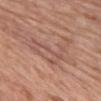<lesion>
<biopsy_status>not biopsied; imaged during a skin examination</biopsy_status>
<lighting>white-light</lighting>
<site>front of the torso</site>
<image>
  <source>total-body photography crop</source>
  <field_of_view_mm>15</field_of_view_mm>
</image>
<lesion_size>
  <long_diameter_mm_approx>7.5</long_diameter_mm_approx>
</lesion_size>
<automated_metrics>
  <vs_skin_darker_L>7.0</vs_skin_darker_L>
  <vs_skin_contrast_norm>5.0</vs_skin_contrast_norm>
  <lesion_detection_confidence_0_100>50</lesion_detection_confidence_0_100>
</automated_metrics>
<patient>
  <sex>male</sex>
  <age_approx>55</age_approx>
</patient>
</lesion>